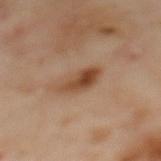Part of a total-body skin-imaging series; this lesion was reviewed on a skin check and was not flagged for biopsy. Cropped from a whole-body photographic skin survey; the tile spans about 15 mm. Located on the upper back. The lesion's longest dimension is about 4.5 mm. A female subject aged approximately 60. Captured under cross-polarized illumination.